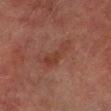follow-up = imaged on a skin check; not biopsied | site = the left lower leg | patient = male, aged around 75 | image = ~15 mm tile from a whole-body skin photo | illumination = cross-polarized illumination | lesion diameter = ≈4.5 mm.